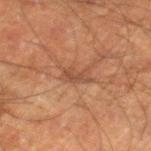{
  "biopsy_status": "not biopsied; imaged during a skin examination",
  "site": "left lower leg",
  "image": {
    "source": "total-body photography crop",
    "field_of_view_mm": 15
  },
  "patient": {
    "sex": "male",
    "age_approx": 50
  }
}